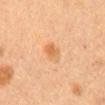Q: Was a biopsy performed?
A: total-body-photography surveillance lesion; no biopsy
Q: What kind of image is this?
A: 15 mm crop, total-body photography
Q: How was the tile lit?
A: cross-polarized
Q: Who is the patient?
A: female, aged approximately 60
Q: Where on the body is the lesion?
A: the mid back
Q: How large is the lesion?
A: ~3 mm (longest diameter)
Q: What did automated image analysis measure?
A: a lesion area of about 4.5 mm² and an outline eccentricity of about 0.85 (0 = round, 1 = elongated); an automated nevus-likeness rating near 70 out of 100 and lesion-presence confidence of about 100/100